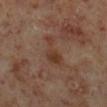Case summary:
• biopsy status — total-body-photography surveillance lesion; no biopsy
• automated metrics — a shape eccentricity near 0.85 and a shape-asymmetry score of about 0.45 (0 = symmetric)
• imaging modality — ~15 mm crop, total-body skin-cancer survey
• anatomic site — the right lower leg
• patient — male, aged around 60